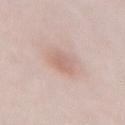Impression: Recorded during total-body skin imaging; not selected for excision or biopsy. Image and clinical context: The lesion-visualizer software estimated a lesion color around L≈64 a*≈18 b*≈25 in CIELAB, roughly 7 lightness units darker than nearby skin, and a normalized lesion–skin contrast near 5. The software also gave a border-irregularity index near 2.5/10, a color-variation rating of about 2/10, and a peripheral color-asymmetry measure near 0.5. The tile uses white-light illumination. The recorded lesion diameter is about 2.5 mm. The lesion is located on the abdomen. A region of skin cropped from a whole-body photographic capture, roughly 15 mm wide. A female patient, aged around 55.